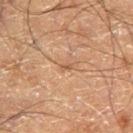Findings:
- biopsy status — total-body-photography surveillance lesion; no biopsy
- body site — the left thigh
- image source — ~15 mm crop, total-body skin-cancer survey
- subject — male, in their 60s